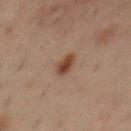Clinical impression:
This lesion was catalogued during total-body skin photography and was not selected for biopsy.
Acquisition and patient details:
Located on the front of the torso. A male subject about 50 years old. Automated image analysis of the tile measured an area of roughly 4 mm², an outline eccentricity of about 0.75 (0 = round, 1 = elongated), and two-axis asymmetry of about 0.2. It also reported an average lesion color of about L≈32 a*≈16 b*≈23 (CIELAB), roughly 9 lightness units darker than nearby skin, and a lesion-to-skin contrast of about 9.5 (normalized; higher = more distinct). The analysis additionally found an automated nevus-likeness rating near 100 out of 100 and a detector confidence of about 100 out of 100 that the crop contains a lesion. Cropped from a whole-body photographic skin survey; the tile spans about 15 mm.